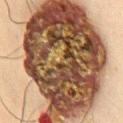Assessment:
The lesion was tiled from a total-body skin photograph and was not biopsied.
Background:
A male patient, aged approximately 35. On the chest. A close-up tile cropped from a whole-body skin photograph, about 15 mm across. An algorithmic analysis of the crop reported a lesion area of about 150 mm², an outline eccentricity of about 0.8 (0 = round, 1 = elongated), and two-axis asymmetry of about 0.2. The software also gave an average lesion color of about L≈46 a*≈17 b*≈29 (CIELAB) and a lesion–skin lightness drop of about 23. The analysis additionally found a border-irregularity index near 3/10, a within-lesion color-variation index near 10/10, and radial color variation of about 7. Longest diameter approximately 18 mm. Captured under cross-polarized illumination.